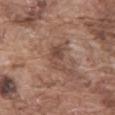Impression: The lesion was tiled from a total-body skin photograph and was not biopsied. Background: A male subject aged 73–77. The tile uses white-light illumination. A roughly 15 mm field-of-view crop from a total-body skin photograph. On the abdomen.